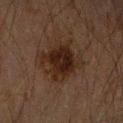biopsy status: total-body-photography surveillance lesion; no biopsy
image source: ~15 mm tile from a whole-body skin photo
anatomic site: the arm
image-analysis metrics: an area of roughly 19 mm², a shape eccentricity near 0.55, and a shape-asymmetry score of about 0.25 (0 = symmetric)
lighting: cross-polarized
subject: male, about 60 years old